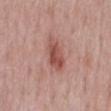patient: male, aged 48–52
anatomic site: the mid back
illumination: white-light illumination
image: 15 mm crop, total-body photography
size: ≈4 mm